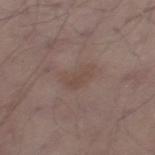| key | value |
|---|---|
| workup | catalogued during a skin exam; not biopsied |
| patient | male, aged approximately 55 |
| anatomic site | the left thigh |
| imaging modality | ~15 mm tile from a whole-body skin photo |